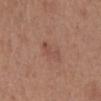Clinical impression:
Part of a total-body skin-imaging series; this lesion was reviewed on a skin check and was not flagged for biopsy.
Image and clinical context:
The total-body-photography lesion software estimated a lesion color around L≈49 a*≈22 b*≈27 in CIELAB, about 6 CIELAB-L* units darker than the surrounding skin, and a normalized lesion–skin contrast near 4.5. It also reported a border-irregularity index near 4.5/10, internal color variation of about 0.5 on a 0–10 scale, and peripheral color asymmetry of about 0. On the front of the torso. A 15 mm close-up tile from a total-body photography series done for melanoma screening. Measured at roughly 2.5 mm in maximum diameter. A male patient aged 63–67.